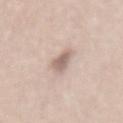Notes:
- automated lesion analysis — roughly 12 lightness units darker than nearby skin; border irregularity of about 3.5 on a 0–10 scale, a within-lesion color-variation index near 2/10, and peripheral color asymmetry of about 0.5
- imaging modality — 15 mm crop, total-body photography
- subject — male, in their mid-40s
- anatomic site — the lower back
- illumination — white-light illumination